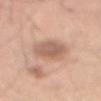notes — imaged on a skin check; not biopsied | site — the front of the torso | illumination — white-light | image source — total-body-photography crop, ~15 mm field of view | subject — male, approximately 50 years of age | automated lesion analysis — a lesion area of about 11 mm² and a shape-asymmetry score of about 0.2 (0 = symmetric); about 11 CIELAB-L* units darker than the surrounding skin and a normalized lesion–skin contrast near 7; border irregularity of about 2 on a 0–10 scale, a within-lesion color-variation index near 3.5/10, and peripheral color asymmetry of about 1 | lesion diameter — ≈4.5 mm.